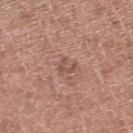{
  "biopsy_status": "not biopsied; imaged during a skin examination",
  "automated_metrics": {
    "area_mm2_approx": 3.5,
    "eccentricity": 0.7,
    "shape_asymmetry": 0.4,
    "cielab_L": 52,
    "cielab_a": 23,
    "cielab_b": 24,
    "vs_skin_darker_L": 8.0,
    "vs_skin_contrast_norm": 5.5
  },
  "image": {
    "source": "total-body photography crop",
    "field_of_view_mm": 15
  },
  "lighting": "white-light",
  "lesion_size": {
    "long_diameter_mm_approx": 2.5
  },
  "patient": {
    "sex": "male",
    "age_approx": 65
  },
  "site": "leg"
}